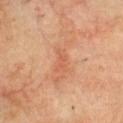acquisition = 15 mm crop, total-body photography | patient = male, aged 68–72 | location = the chest.